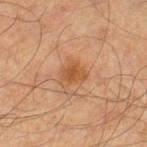Q: Is there a histopathology result?
A: catalogued during a skin exam; not biopsied
Q: What is the lesion's diameter?
A: ≈3 mm
Q: Where on the body is the lesion?
A: the left lower leg
Q: Who is the patient?
A: male, in their 60s
Q: Illumination type?
A: cross-polarized
Q: How was this image acquired?
A: ~15 mm tile from a whole-body skin photo